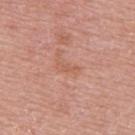{"biopsy_status": "not biopsied; imaged during a skin examination", "image": {"source": "total-body photography crop", "field_of_view_mm": 15}, "lighting": "white-light", "lesion_size": {"long_diameter_mm_approx": 2.5}, "patient": {"sex": "male", "age_approx": 70}, "automated_metrics": {"area_mm2_approx": 2.5, "eccentricity": 0.9, "shape_asymmetry": 0.35, "border_irregularity_0_10": 4.0, "color_variation_0_10": 0.0, "nevus_likeness_0_100": 0}, "site": "upper back"}